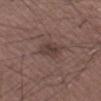follow-up = no biopsy performed (imaged during a skin exam); patient = male, aged around 75; anatomic site = the left thigh; image source = ~15 mm crop, total-body skin-cancer survey; diameter = about 3 mm; illumination = white-light illumination.